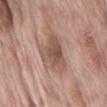workup — no biopsy performed (imaged during a skin exam); anatomic site — the abdomen; lesion size — about 5.5 mm; lighting — white-light; image — ~15 mm tile from a whole-body skin photo; patient — male, roughly 70 years of age.